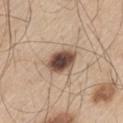workup = imaged on a skin check; not biopsied
location = the left thigh
image = ~15 mm crop, total-body skin-cancer survey
patient = male, about 60 years old